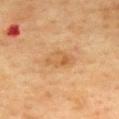Imaged during a routine full-body skin examination; the lesion was not biopsied and no histopathology is available. Cropped from a total-body skin-imaging series; the visible field is about 15 mm. A female subject in their mid- to late 50s. Captured under cross-polarized illumination. Located on the mid back. Approximately 4 mm at its widest. Automated tile analysis of the lesion measured an area of roughly 6.5 mm², an eccentricity of roughly 0.9, and two-axis asymmetry of about 0.25. And it measured an average lesion color of about L≈53 a*≈19 b*≈37 (CIELAB) and a normalized border contrast of about 5.5. And it measured a border-irregularity index near 3/10, internal color variation of about 4.5 on a 0–10 scale, and radial color variation of about 1.5. The analysis additionally found an automated nevus-likeness rating near 0 out of 100 and lesion-presence confidence of about 100/100.